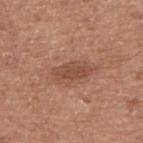TBP lesion metrics — a lesion area of about 7 mm² and a symmetry-axis asymmetry near 0.25; an automated nevus-likeness rating near 60 out of 100 and a lesion-detection confidence of about 100/100
patient — male, aged approximately 65
lesion diameter — ≈4 mm
tile lighting — white-light illumination
imaging modality — ~15 mm tile from a whole-body skin photo
location — the right upper arm